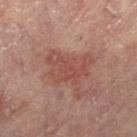Captured during whole-body skin photography for melanoma surveillance; the lesion was not biopsied.
Located on the left leg.
A female patient roughly 80 years of age.
The lesion's longest dimension is about 5.5 mm.
A 15 mm crop from a total-body photograph taken for skin-cancer surveillance.
Imaged with cross-polarized lighting.